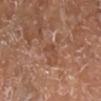The lesion was photographed on a routine skin check and not biopsied; there is no pathology result. A female patient about 75 years old. A 15 mm close-up tile from a total-body photography series done for melanoma screening. From the right lower leg. The lesion's longest dimension is about 2.5 mm.